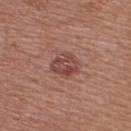workup: no biopsy performed (imaged during a skin exam)